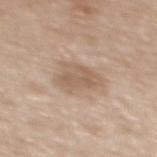Captured during whole-body skin photography for melanoma surveillance; the lesion was not biopsied. Longest diameter approximately 5.5 mm. This image is a 15 mm lesion crop taken from a total-body photograph. The lesion is located on the upper back. A female subject, about 65 years old.